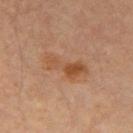| feature | finding |
|---|---|
| follow-up | imaged on a skin check; not biopsied |
| acquisition | ~15 mm crop, total-body skin-cancer survey |
| subject | male, about 65 years old |
| location | the right upper arm |